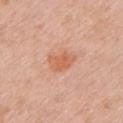biopsy status = no biopsy performed (imaged during a skin exam); size = about 3.5 mm; body site = the left upper arm; acquisition = total-body-photography crop, ~15 mm field of view; lighting = white-light illumination; patient = female, aged 38 to 42.